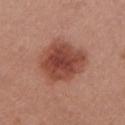Q: Was a biopsy performed?
A: catalogued during a skin exam; not biopsied
Q: Where on the body is the lesion?
A: the front of the torso
Q: Automated lesion metrics?
A: an area of roughly 23 mm², a shape eccentricity near 0.5, and a shape-asymmetry score of about 0.15 (0 = symmetric); a mean CIELAB color near L≈46 a*≈26 b*≈29, a lesion–skin lightness drop of about 13, and a lesion-to-skin contrast of about 9 (normalized; higher = more distinct)
Q: What is the lesion's diameter?
A: ~6 mm (longest diameter)
Q: What kind of image is this?
A: ~15 mm crop, total-body skin-cancer survey
Q: Patient demographics?
A: female, roughly 25 years of age
Q: Illumination type?
A: white-light illumination